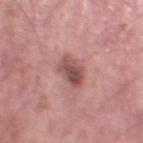No biopsy was performed on this lesion — it was imaged during a full skin examination and was not determined to be concerning. A region of skin cropped from a whole-body photographic capture, roughly 15 mm wide. An algorithmic analysis of the crop reported a lesion area of about 7.5 mm², an eccentricity of roughly 0.7, and a shape-asymmetry score of about 0.2 (0 = symmetric). The software also gave an automated nevus-likeness rating near 45 out of 100 and a detector confidence of about 100 out of 100 that the crop contains a lesion. The tile uses white-light illumination. A male patient, in their mid-50s. From the leg. Longest diameter approximately 3.5 mm.